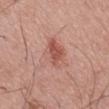biopsy status: imaged on a skin check; not biopsied
subject: male, roughly 55 years of age
lesion size: ~5.5 mm (longest diameter)
image: 15 mm crop, total-body photography
body site: the mid back